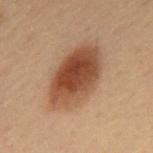Assessment:
The lesion was photographed on a routine skin check and not biopsied; there is no pathology result.
Clinical summary:
Captured under cross-polarized illumination. From the mid back. This image is a 15 mm lesion crop taken from a total-body photograph. A male patient in their mid- to late 50s.